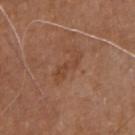<record>
  <biopsy_status>not biopsied; imaged during a skin examination</biopsy_status>
  <automated_metrics>
    <area_mm2_approx>5.0</area_mm2_approx>
    <eccentricity>0.95</eccentricity>
    <shape_asymmetry>0.4</shape_asymmetry>
    <border_irregularity_0_10>5.5</border_irregularity_0_10>
    <color_variation_0_10>0.5</color_variation_0_10>
    <peripheral_color_asymmetry>0.0</peripheral_color_asymmetry>
    <nevus_likeness_0_100>0</nevus_likeness_0_100>
    <lesion_detection_confidence_0_100>100</lesion_detection_confidence_0_100>
  </automated_metrics>
  <lesion_size>
    <long_diameter_mm_approx>4.5</long_diameter_mm_approx>
  </lesion_size>
  <site>chest</site>
  <lighting>white-light</lighting>
  <image>
    <source>total-body photography crop</source>
    <field_of_view_mm>15</field_of_view_mm>
  </image>
  <patient>
    <sex>male</sex>
    <age_approx>70</age_approx>
  </patient>
</record>